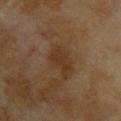This lesion was catalogued during total-body skin photography and was not selected for biopsy. A female patient aged around 60. Located on the upper back. The recorded lesion diameter is about 3.5 mm. A 15 mm close-up extracted from a 3D total-body photography capture.